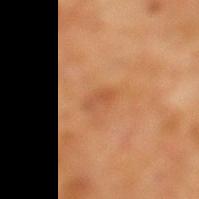Case summary:
• follow-up — catalogued during a skin exam; not biopsied
• acquisition — ~15 mm crop, total-body skin-cancer survey
• subject — male, aged approximately 60
• anatomic site — the leg
• lighting — cross-polarized
• image-analysis metrics — a nevus-likeness score of about 5/100 and a lesion-detection confidence of about 100/100
• lesion size — about 2.5 mm